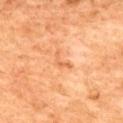Captured during whole-body skin photography for melanoma surveillance; the lesion was not biopsied. The subject is a female aged approximately 60. A roughly 15 mm field-of-view crop from a total-body skin photograph. Longest diameter approximately 3 mm. The tile uses cross-polarized illumination. Located on the upper back.